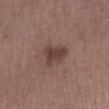Captured during whole-body skin photography for melanoma surveillance; the lesion was not biopsied.
A male patient in their mid- to late 50s.
A lesion tile, about 15 mm wide, cut from a 3D total-body photograph.
The lesion's longest dimension is about 3.5 mm.
The total-body-photography lesion software estimated border irregularity of about 4 on a 0–10 scale and a color-variation rating of about 2.5/10.
Imaged with white-light lighting.
Located on the left lower leg.